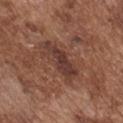<lesion>
  <biopsy_status>not biopsied; imaged during a skin examination</biopsy_status>
  <lighting>white-light</lighting>
  <image>
    <source>total-body photography crop</source>
    <field_of_view_mm>15</field_of_view_mm>
  </image>
  <site>chest</site>
  <automated_metrics>
    <border_irregularity_0_10>5.0</border_irregularity_0_10>
    <color_variation_0_10>2.0</color_variation_0_10>
    <peripheral_color_asymmetry>0.5</peripheral_color_asymmetry>
    <nevus_likeness_0_100>0</nevus_likeness_0_100>
  </automated_metrics>
  <patient>
    <sex>male</sex>
    <age_approx>75</age_approx>
  </patient>
  <lesion_size>
    <long_diameter_mm_approx>5.5</long_diameter_mm_approx>
  </lesion_size>
</lesion>